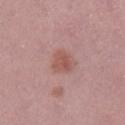- follow-up: no biopsy performed (imaged during a skin exam)
- image-analysis metrics: a nevus-likeness score of about 45/100 and lesion-presence confidence of about 100/100
- body site: the left lower leg
- patient: female, aged 13–17
- image source: ~15 mm crop, total-body skin-cancer survey
- size: about 3 mm